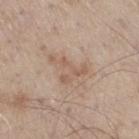Recorded during total-body skin imaging; not selected for excision or biopsy.
A male patient aged 58–62.
Located on the right thigh.
A roughly 15 mm field-of-view crop from a total-body skin photograph.